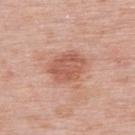| key | value |
|---|---|
| notes | no biopsy performed (imaged during a skin exam) |
| TBP lesion metrics | internal color variation of about 3 on a 0–10 scale and a peripheral color-asymmetry measure near 1; an automated nevus-likeness rating near 55 out of 100 |
| image source | ~15 mm crop, total-body skin-cancer survey |
| subject | female, aged approximately 50 |
| size | about 4.5 mm |
| illumination | white-light illumination |
| site | the back |